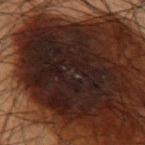<lesion>
<patient>
  <sex>male</sex>
  <age_approx>50</age_approx>
</patient>
<lighting>cross-polarized</lighting>
<site>left upper arm</site>
<lesion_size>
  <long_diameter_mm_approx>14.0</long_diameter_mm_approx>
</lesion_size>
<automated_metrics>
  <cielab_L>14</cielab_L>
  <cielab_a>13</cielab_a>
  <cielab_b>14</cielab_b>
  <vs_skin_darker_L>11.0</vs_skin_darker_L>
  <vs_skin_contrast_norm>17.0</vs_skin_contrast_norm>
  <lesion_detection_confidence_0_100>0</lesion_detection_confidence_0_100>
</automated_metrics>
<image>
  <source>total-body photography crop</source>
  <field_of_view_mm>15</field_of_view_mm>
</image>
<diagnosis>
  <histopathology>invasive melanoma</histopathology>
  <malignancy>malignant</malignancy>
  <taxonomic_path>Malignant, Malignant melanocytic proliferations (Melanoma), Melanoma Invasive</taxonomic_path>
  <breslow_mm>0.5</breslow_mm>
  <mitotic_index>0/mm²</mitotic_index>
</diagnosis>
</lesion>